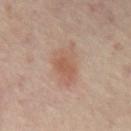Imaged with cross-polarized lighting.
A 15 mm crop from a total-body photograph taken for skin-cancer surveillance.
Measured at roughly 5.5 mm in maximum diameter.
A patient approximately 55 years of age.
The total-body-photography lesion software estimated an average lesion color of about L≈55 a*≈19 b*≈28 (CIELAB) and a normalized lesion–skin contrast near 6. And it measured a border-irregularity rating of about 4.5/10 and radial color variation of about 1. It also reported a classifier nevus-likeness of about 35/100 and a detector confidence of about 100 out of 100 that the crop contains a lesion.
The lesion is located on the mid back.